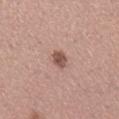workup: catalogued during a skin exam; not biopsied
location: the right lower leg
subject: female, aged approximately 30
imaging modality: ~15 mm crop, total-body skin-cancer survey
lighting: white-light
lesion size: about 2.5 mm